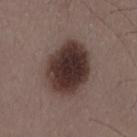Assessment:
No biopsy was performed on this lesion — it was imaged during a full skin examination and was not determined to be concerning.
Image and clinical context:
Automated tile analysis of the lesion measured an area of roughly 25 mm² and an outline eccentricity of about 0.7 (0 = round, 1 = elongated). It also reported a mean CIELAB color near L≈32 a*≈15 b*≈18, roughly 17 lightness units darker than nearby skin, and a lesion-to-skin contrast of about 14 (normalized; higher = more distinct). Captured under white-light illumination. A male patient about 50 years old. Located on the lower back. A 15 mm close-up tile from a total-body photography series done for melanoma screening.